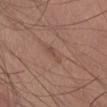notes: catalogued during a skin exam; not biopsied | subject: male, approximately 80 years of age | location: the abdomen | diameter: about 3 mm | imaging modality: ~15 mm tile from a whole-body skin photo.